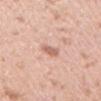The lesion was tiled from a total-body skin photograph and was not biopsied. The recorded lesion diameter is about 2.5 mm. A female subject, approximately 40 years of age. Cropped from a total-body skin-imaging series; the visible field is about 15 mm. The lesion is on the right upper arm.